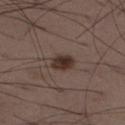follow-up=no biopsy performed (imaged during a skin exam)
image source=15 mm crop, total-body photography
automated metrics=a footprint of about 6 mm²; a normalized lesion–skin contrast near 10; a classifier nevus-likeness of about 100/100 and lesion-presence confidence of about 100/100
location=the leg
lighting=white-light
patient=male, aged approximately 50
lesion size=≈3 mm